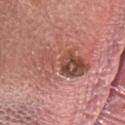Context: On the head or neck. Automated image analysis of the tile measured a border-irregularity index near 7/10, internal color variation of about 9.5 on a 0–10 scale, and peripheral color asymmetry of about 4.5. The software also gave a nevus-likeness score of about 0/100. Captured under white-light illumination. The patient is a female in their mid- to late 40s. A region of skin cropped from a whole-body photographic capture, roughly 15 mm wide.